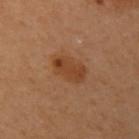Imaged during a routine full-body skin examination; the lesion was not biopsied and no histopathology is available. About 4 mm across. Cropped from a total-body skin-imaging series; the visible field is about 15 mm. This is a cross-polarized tile. The patient is a female in their 60s. The lesion is located on the arm. Automated image analysis of the tile measured a footprint of about 9 mm². It also reported roughly 7 lightness units darker than nearby skin and a normalized lesion–skin contrast near 7. The analysis additionally found a border-irregularity index near 2/10, internal color variation of about 3 on a 0–10 scale, and peripheral color asymmetry of about 1.